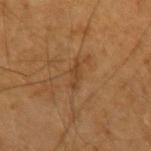Clinical impression:
Recorded during total-body skin imaging; not selected for excision or biopsy.
Context:
This is a cross-polarized tile. The total-body-photography lesion software estimated an area of roughly 3.5 mm². And it measured a lesion–skin lightness drop of about 6. It also reported a border-irregularity rating of about 4/10 and radial color variation of about 0. The lesion's longest dimension is about 3 mm. The patient is a male in their 60s. The lesion is located on the right upper arm. A close-up tile cropped from a whole-body skin photograph, about 15 mm across.